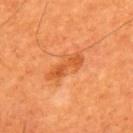Impression:
The lesion was photographed on a routine skin check and not biopsied; there is no pathology result.
Background:
Located on the upper back. A male subject roughly 60 years of age. Cropped from a whole-body photographic skin survey; the tile spans about 15 mm. Captured under cross-polarized illumination.